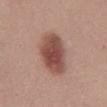{"biopsy_status": "not biopsied; imaged during a skin examination", "patient": {"sex": "female", "age_approx": 25}, "image": {"source": "total-body photography crop", "field_of_view_mm": 15}, "site": "mid back"}